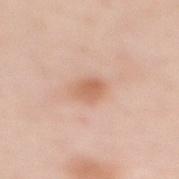Clinical impression:
This lesion was catalogued during total-body skin photography and was not selected for biopsy.
Acquisition and patient details:
A 15 mm crop from a total-body photograph taken for skin-cancer surveillance. A female subject aged approximately 50. From the mid back.